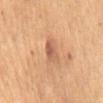{"biopsy_status": "not biopsied; imaged during a skin examination", "image": {"source": "total-body photography crop", "field_of_view_mm": 15}, "patient": {"sex": "female", "age_approx": 80}, "lighting": "cross-polarized", "site": "abdomen", "lesion_size": {"long_diameter_mm_approx": 3.0}, "automated_metrics": {"area_mm2_approx": 3.5, "shape_asymmetry": 0.3}}